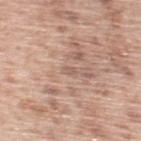Assessment:
Recorded during total-body skin imaging; not selected for excision or biopsy.
Background:
A 15 mm crop from a total-body photograph taken for skin-cancer surveillance. On the upper back. The lesion's longest dimension is about 1 mm. A male patient aged around 55. The total-body-photography lesion software estimated a footprint of about 1 mm² and an eccentricity of roughly 0.7. The software also gave a nevus-likeness score of about 0/100.